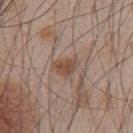{"biopsy_status": "not biopsied; imaged during a skin examination", "patient": {"sex": "male", "age_approx": 45}, "lesion_size": {"long_diameter_mm_approx": 3.0}, "image": {"source": "total-body photography crop", "field_of_view_mm": 15}, "lighting": "white-light", "site": "front of the torso"}